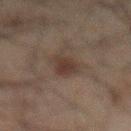Case summary:
– image · ~15 mm tile from a whole-body skin photo
– illumination · cross-polarized illumination
– body site · the abdomen
– lesion size · ~3.5 mm (longest diameter)
– subject · male, about 60 years old